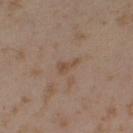Findings:
- follow-up — no biopsy performed (imaged during a skin exam)
- acquisition — ~15 mm tile from a whole-body skin photo
- body site — the right upper arm
- size — about 2.5 mm
- illumination — cross-polarized
- automated lesion analysis — an area of roughly 2.5 mm² and a symmetry-axis asymmetry near 0.55; a border-irregularity rating of about 5.5/10 and a color-variation rating of about 0/10; a classifier nevus-likeness of about 0/100
- patient — male, roughly 55 years of age